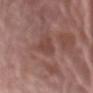* workup — total-body-photography surveillance lesion; no biopsy
* location — the front of the torso
* image-analysis metrics — an area of roughly 5.5 mm², an eccentricity of roughly 0.65, and a shape-asymmetry score of about 0.35 (0 = symmetric); roughly 7 lightness units darker than nearby skin and a lesion-to-skin contrast of about 6 (normalized; higher = more distinct); border irregularity of about 3.5 on a 0–10 scale, a color-variation rating of about 1.5/10, and a peripheral color-asymmetry measure near 0.5
* imaging modality — total-body-photography crop, ~15 mm field of view
* tile lighting — white-light
* subject — female, in their mid- to late 70s